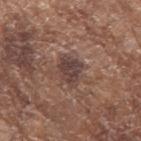{
  "biopsy_status": "not biopsied; imaged during a skin examination",
  "site": "left forearm",
  "lighting": "white-light",
  "lesion_size": {
    "long_diameter_mm_approx": 3.5
  },
  "patient": {
    "sex": "female",
    "age_approx": 75
  },
  "image": {
    "source": "total-body photography crop",
    "field_of_view_mm": 15
  }
}